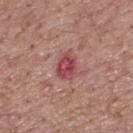Clinical impression: Part of a total-body skin-imaging series; this lesion was reviewed on a skin check and was not flagged for biopsy. Context: The lesion is on the upper back. A close-up tile cropped from a whole-body skin photograph, about 15 mm across. A male subject, approximately 70 years of age.